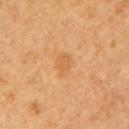biopsy_status: not biopsied; imaged during a skin examination
lighting: cross-polarized
image:
  source: total-body photography crop
  field_of_view_mm: 15
patient:
  sex: female
  age_approx: 60
lesion_size:
  long_diameter_mm_approx: 2.5
site: left upper arm
automated_metrics:
  cielab_L: 58
  cielab_a: 22
  cielab_b: 41
  vs_skin_darker_L: 6.0